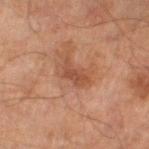| key | value |
|---|---|
| imaging modality | 15 mm crop, total-body photography |
| subject | male, aged 68 to 72 |
| lesion size | ≈3.5 mm |
| location | the leg |
| lighting | cross-polarized illumination |